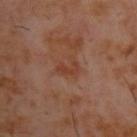Recorded during total-body skin imaging; not selected for excision or biopsy. This is a cross-polarized tile. Automated tile analysis of the lesion measured a footprint of about 4 mm², an eccentricity of roughly 0.8, and a shape-asymmetry score of about 0.35 (0 = symmetric). A 15 mm close-up extracted from a 3D total-body photography capture. Longest diameter approximately 3 mm. A male patient roughly 60 years of age. The lesion is located on the upper back.